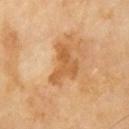Case summary:
• body site — the right upper arm
• subject — male, in their mid- to late 60s
• image source — 15 mm crop, total-body photography